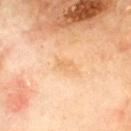Clinical impression:
The lesion was tiled from a total-body skin photograph and was not biopsied.
Context:
An algorithmic analysis of the crop reported an average lesion color of about L≈71 a*≈20 b*≈42 (CIELAB) and a normalized lesion–skin contrast near 5. The software also gave internal color variation of about 1 on a 0–10 scale and radial color variation of about 0.5. The recorded lesion diameter is about 3 mm. A male subject about 70 years old. Cropped from a whole-body photographic skin survey; the tile spans about 15 mm. From the mid back.